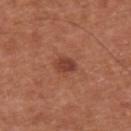follow-up: imaged on a skin check; not biopsied
lighting: white-light illumination
lesion diameter: ~2.5 mm (longest diameter)
anatomic site: the upper back
image-analysis metrics: an area of roughly 4 mm² and an eccentricity of roughly 0.8; an average lesion color of about L≈42 a*≈26 b*≈29 (CIELAB), roughly 10 lightness units darker than nearby skin, and a lesion-to-skin contrast of about 8 (normalized; higher = more distinct); a border-irregularity rating of about 2/10, internal color variation of about 2.5 on a 0–10 scale, and a peripheral color-asymmetry measure near 0.5; a classifier nevus-likeness of about 90/100 and a detector confidence of about 100 out of 100 that the crop contains a lesion
subject: male, aged 63 to 67
image source: 15 mm crop, total-body photography